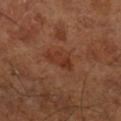Q: How large is the lesion?
A: ≈4 mm
Q: What lighting was used for the tile?
A: cross-polarized
Q: What is the anatomic site?
A: the right lower leg
Q: How was this image acquired?
A: ~15 mm tile from a whole-body skin photo
Q: What are the patient's age and sex?
A: in their mid-60s
Q: Automated lesion metrics?
A: an area of roughly 6 mm² and a symmetry-axis asymmetry near 0.4; an average lesion color of about L≈34 a*≈24 b*≈30 (CIELAB) and a normalized lesion–skin contrast near 6